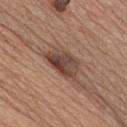Captured during whole-body skin photography for melanoma surveillance; the lesion was not biopsied. From the chest. Approximately 5 mm at its widest. A roughly 15 mm field-of-view crop from a total-body skin photograph. The lesion-visualizer software estimated a mean CIELAB color near L≈44 a*≈18 b*≈25, about 12 CIELAB-L* units darker than the surrounding skin, and a lesion-to-skin contrast of about 9.5 (normalized; higher = more distinct). The software also gave border irregularity of about 3 on a 0–10 scale, internal color variation of about 6.5 on a 0–10 scale, and radial color variation of about 2.5. The analysis additionally found a classifier nevus-likeness of about 35/100. A male patient, aged approximately 65.